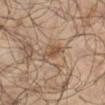{
  "biopsy_status": "not biopsied; imaged during a skin examination",
  "automated_metrics": {
    "area_mm2_approx": 5.0,
    "eccentricity": 0.85,
    "shape_asymmetry": 0.4,
    "cielab_L": 48,
    "cielab_a": 16,
    "cielab_b": 28,
    "vs_skin_darker_L": 8.0
  },
  "lighting": "cross-polarized",
  "image": {
    "source": "total-body photography crop",
    "field_of_view_mm": 15
  },
  "site": "right lower leg",
  "lesion_size": {
    "long_diameter_mm_approx": 3.5
  },
  "patient": {
    "sex": "male",
    "age_approx": 65
  }
}